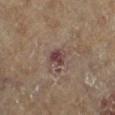biopsy_status: not biopsied; imaged during a skin examination
site: right lower leg
lesion_size:
  long_diameter_mm_approx: 3.0
image:
  source: total-body photography crop
  field_of_view_mm: 15
patient:
  sex: male
  age_approx: 85+